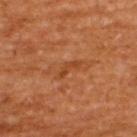biopsy_status: not biopsied; imaged during a skin examination
site: upper back
patient:
  sex: male
  age_approx: 65
lesion_size:
  long_diameter_mm_approx: 3.0
lighting: cross-polarized
automated_metrics:
  cielab_L: 42
  cielab_a: 27
  cielab_b: 38
  vs_skin_darker_L: 7.0
  vs_skin_contrast_norm: 6.0
  border_irregularity_0_10: 5.5
  peripheral_color_asymmetry: 0.0
image:
  source: total-body photography crop
  field_of_view_mm: 15